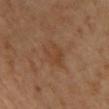| field | value |
|---|---|
| notes | imaged on a skin check; not biopsied |
| image source | 15 mm crop, total-body photography |
| tile lighting | cross-polarized illumination |
| patient | male, aged 53 to 57 |
| body site | the chest |